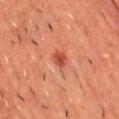- follow-up: imaged on a skin check; not biopsied
- image-analysis metrics: a shape eccentricity near 0.6 and two-axis asymmetry of about 0.25; a mean CIELAB color near L≈49 a*≈35 b*≈35, about 11 CIELAB-L* units darker than the surrounding skin, and a normalized border contrast of about 7.5; lesion-presence confidence of about 100/100
- acquisition: total-body-photography crop, ~15 mm field of view
- lighting: cross-polarized
- anatomic site: the chest
- subject: male, in their mid-40s